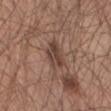notes = catalogued during a skin exam; not biopsied
lesion size = ≈4 mm
acquisition = ~15 mm crop, total-body skin-cancer survey
illumination = white-light
TBP lesion metrics = a lesion color around L≈42 a*≈17 b*≈24 in CIELAB, roughly 10 lightness units darker than nearby skin, and a normalized lesion–skin contrast near 8; border irregularity of about 2.5 on a 0–10 scale, a within-lesion color-variation index near 3.5/10, and a peripheral color-asymmetry measure near 1.5
patient = male, in their mid- to late 60s
anatomic site = the front of the torso